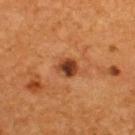This lesion was catalogued during total-body skin photography and was not selected for biopsy. Located on the upper back. A lesion tile, about 15 mm wide, cut from a 3D total-body photograph. Captured under cross-polarized illumination. Approximately 2.5 mm at its widest. A male patient, aged around 55.